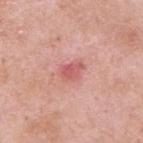This lesion was catalogued during total-body skin photography and was not selected for biopsy.
The lesion is on the upper back.
A roughly 15 mm field-of-view crop from a total-body skin photograph.
A male subject, aged 53–57.